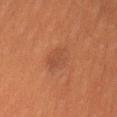| key | value |
|---|---|
| workup | catalogued during a skin exam; not biopsied |
| subject | female, in their 30s |
| imaging modality | total-body-photography crop, ~15 mm field of view |
| tile lighting | cross-polarized |
| anatomic site | the left leg |